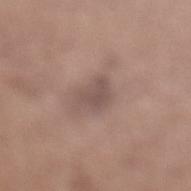Captured during whole-body skin photography for melanoma surveillance; the lesion was not biopsied. This is a white-light tile. From the left lower leg. Automated tile analysis of the lesion measured a mean CIELAB color near L≈50 a*≈16 b*≈21 and about 8 CIELAB-L* units darker than the surrounding skin. The software also gave radial color variation of about 0.5. And it measured a nevus-likeness score of about 0/100 and lesion-presence confidence of about 60/100. A region of skin cropped from a whole-body photographic capture, roughly 15 mm wide. A female subject aged around 55. Approximately 3 mm at its widest.